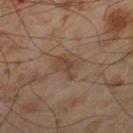biopsy_status: not biopsied; imaged during a skin examination
automated_metrics:
  color_variation_0_10: 4.0
  peripheral_color_asymmetry: 1.0
  nevus_likeness_0_100: 0
  lesion_detection_confidence_0_100: 100
image:
  source: total-body photography crop
  field_of_view_mm: 15
patient:
  sex: male
  age_approx: 55
site: left thigh
lighting: cross-polarized